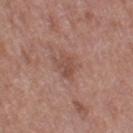notes = catalogued during a skin exam; not biopsied
subject = female, aged approximately 40
diameter = ≈2.5 mm
site = the left thigh
acquisition = ~15 mm tile from a whole-body skin photo